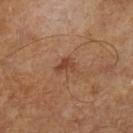Assessment:
Part of a total-body skin-imaging series; this lesion was reviewed on a skin check and was not flagged for biopsy.
Context:
About 2.5 mm across. The subject is a male aged 63–67. Imaged with cross-polarized lighting. Automated tile analysis of the lesion measured a footprint of about 4 mm² and a symmetry-axis asymmetry near 0.4. It also reported a mean CIELAB color near L≈43 a*≈22 b*≈31. A roughly 15 mm field-of-view crop from a total-body skin photograph.